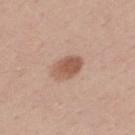The lesion was tiled from a total-body skin photograph and was not biopsied. The tile uses white-light illumination. A male patient, roughly 40 years of age. Approximately 4 mm at its widest. Cropped from a whole-body photographic skin survey; the tile spans about 15 mm. Located on the upper back.